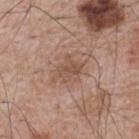Q: Where on the body is the lesion?
A: the upper back
Q: Lesion size?
A: about 3 mm
Q: Who is the patient?
A: male, in their mid-60s
Q: What kind of image is this?
A: 15 mm crop, total-body photography
Q: How was the tile lit?
A: white-light